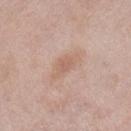Imaged during a routine full-body skin examination; the lesion was not biopsied and no histopathology is available. The lesion is located on the right lower leg. The lesion-visualizer software estimated a lesion area of about 5.5 mm², an outline eccentricity of about 0.85 (0 = round, 1 = elongated), and a symmetry-axis asymmetry near 0.35. The analysis additionally found a mean CIELAB color near L≈61 a*≈19 b*≈28, a lesion–skin lightness drop of about 7, and a lesion-to-skin contrast of about 5.5 (normalized; higher = more distinct). And it measured a classifier nevus-likeness of about 0/100 and a detector confidence of about 100 out of 100 that the crop contains a lesion. A female patient, in their mid- to late 60s. Cropped from a total-body skin-imaging series; the visible field is about 15 mm. The tile uses white-light illumination.